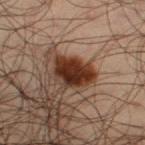biopsy status: no biopsy performed (imaged during a skin exam) | image source: 15 mm crop, total-body photography | site: the right thigh | patient: male, roughly 35 years of age.